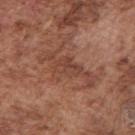Case summary:
* biopsy status — no biopsy performed (imaged during a skin exam)
* patient — male, about 75 years old
* image — ~15 mm tile from a whole-body skin photo
* size — ~7 mm (longest diameter)
* anatomic site — the right upper arm
* tile lighting — white-light illumination